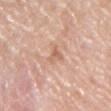The lesion was tiled from a total-body skin photograph and was not biopsied.
The lesion is on the chest.
This is a white-light tile.
This image is a 15 mm lesion crop taken from a total-body photograph.
The recorded lesion diameter is about 3 mm.
The patient is a male aged around 55.